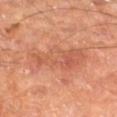This lesion was catalogued during total-body skin photography and was not selected for biopsy. On the left thigh. A male patient, aged 63–67. A roughly 15 mm field-of-view crop from a total-body skin photograph.